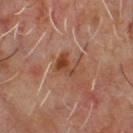workup: total-body-photography surveillance lesion; no biopsy